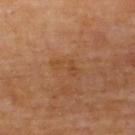Assessment: The lesion was photographed on a routine skin check and not biopsied; there is no pathology result. Acquisition and patient details: Imaged with cross-polarized lighting. This image is a 15 mm lesion crop taken from a total-body photograph. The total-body-photography lesion software estimated a normalized border contrast of about 5. The software also gave border irregularity of about 5.5 on a 0–10 scale, internal color variation of about 1 on a 0–10 scale, and radial color variation of about 0.5. And it measured lesion-presence confidence of about 100/100. From the upper back. Longest diameter approximately 3.5 mm. A male patient, aged 58 to 62.